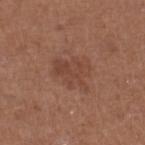workup — catalogued during a skin exam; not biopsied | lesion size — about 4.5 mm | illumination — white-light illumination | acquisition — 15 mm crop, total-body photography | site — the right lower leg | patient — female, aged approximately 40 | image-analysis metrics — a shape eccentricity near 0.6; a lesion-to-skin contrast of about 5 (normalized; higher = more distinct).